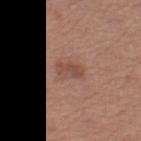workup: no biopsy performed (imaged during a skin exam)
body site: the left thigh
subject: female, aged around 65
diameter: ≈3 mm
illumination: white-light
imaging modality: total-body-photography crop, ~15 mm field of view
automated metrics: a footprint of about 4 mm², an outline eccentricity of about 0.9 (0 = round, 1 = elongated), and two-axis asymmetry of about 0.25; an average lesion color of about L≈48 a*≈22 b*≈27 (CIELAB) and a lesion-to-skin contrast of about 6 (normalized; higher = more distinct)